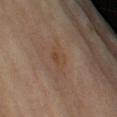The lesion was tiled from a total-body skin photograph and was not biopsied. The subject is a male aged 68 to 72. From the arm. The lesion's longest dimension is about 3.5 mm. The tile uses cross-polarized illumination. A 15 mm close-up tile from a total-body photography series done for melanoma screening.